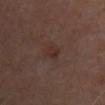<record>
<biopsy_status>not biopsied; imaged during a skin examination</biopsy_status>
<patient>
  <sex>male</sex>
  <age_approx>65</age_approx>
</patient>
<site>mid back</site>
<lesion_size>
  <long_diameter_mm_approx>2.5</long_diameter_mm_approx>
</lesion_size>
<lighting>cross-polarized</lighting>
<image>
  <source>total-body photography crop</source>
  <field_of_view_mm>15</field_of_view_mm>
</image>
</record>